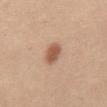biopsy status = total-body-photography surveillance lesion; no biopsy | lesion size = ~3 mm (longest diameter) | location = the abdomen | automated metrics = a lesion color around L≈56 a*≈22 b*≈31 in CIELAB and a normalized lesion–skin contrast near 8.5; a border-irregularity rating of about 1/10, internal color variation of about 2.5 on a 0–10 scale, and a peripheral color-asymmetry measure near 1 | image = total-body-photography crop, ~15 mm field of view | patient = female, aged 43 to 47 | lighting = white-light illumination.